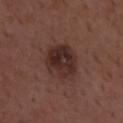Notes:
– automated lesion analysis: a lesion area of about 13 mm² and an outline eccentricity of about 0.55 (0 = round, 1 = elongated); a peripheral color-asymmetry measure near 2; a nevus-likeness score of about 70/100
– subject: male, aged around 50
– lesion diameter: ~4.5 mm (longest diameter)
– imaging modality: ~15 mm tile from a whole-body skin photo
– lighting: white-light